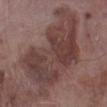biopsy status: no biopsy performed (imaged during a skin exam)
subject: male, aged around 75
image source: total-body-photography crop, ~15 mm field of view
body site: the left lower leg
size: ≈12.5 mm